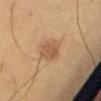Findings:
* follow-up — catalogued during a skin exam; not biopsied
* image — ~15 mm tile from a whole-body skin photo
* anatomic site — the abdomen
* subject — female, aged 48–52
* size — ≈3.5 mm
* automated metrics — a lesion area of about 7 mm² and a shape eccentricity near 0.7; a within-lesion color-variation index near 2.5/10